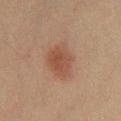No biopsy was performed on this lesion — it was imaged during a full skin examination and was not determined to be concerning. Approximately 4.5 mm at its widest. An algorithmic analysis of the crop reported a lesion color around L≈40 a*≈18 b*≈26 in CIELAB, about 7 CIELAB-L* units darker than the surrounding skin, and a lesion-to-skin contrast of about 6.5 (normalized; higher = more distinct). It also reported a within-lesion color-variation index near 3/10 and radial color variation of about 1. It also reported a nevus-likeness score of about 100/100. The subject is a male aged 58 to 62. Imaged with cross-polarized lighting. The lesion is on the chest. A lesion tile, about 15 mm wide, cut from a 3D total-body photograph.